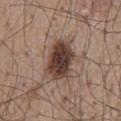follow-up=no biopsy performed (imaged during a skin exam); patient=male, aged around 55; acquisition=15 mm crop, total-body photography; illumination=white-light illumination; lesion diameter=about 5 mm; location=the mid back.